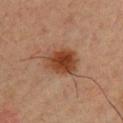Q: Was this lesion biopsied?
A: catalogued during a skin exam; not biopsied
Q: How was the tile lit?
A: cross-polarized illumination
Q: What are the patient's age and sex?
A: male, aged 33–37
Q: What kind of image is this?
A: ~15 mm tile from a whole-body skin photo
Q: Where on the body is the lesion?
A: the chest
Q: What did automated image analysis measure?
A: a mean CIELAB color near L≈39 a*≈22 b*≈30, about 12 CIELAB-L* units darker than the surrounding skin, and a normalized border contrast of about 10; a border-irregularity rating of about 2/10, a within-lesion color-variation index near 5/10, and radial color variation of about 1.5; a nevus-likeness score of about 100/100
Q: How large is the lesion?
A: ≈4.5 mm